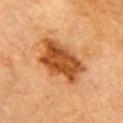{
  "biopsy_status": "not biopsied; imaged during a skin examination",
  "lighting": "cross-polarized",
  "image": {
    "source": "total-body photography crop",
    "field_of_view_mm": 15
  },
  "lesion_size": {
    "long_diameter_mm_approx": 7.0
  },
  "patient": {
    "sex": "male",
    "age_approx": "85+"
  },
  "automated_metrics": {
    "area_mm2_approx": 20.0,
    "eccentricity": 0.8,
    "shape_asymmetry": 0.2,
    "border_irregularity_0_10": 3.0,
    "color_variation_0_10": 4.0,
    "nevus_likeness_0_100": 70,
    "lesion_detection_confidence_0_100": 100
  },
  "site": "chest"
}